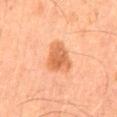Captured during whole-body skin photography for melanoma surveillance; the lesion was not biopsied.